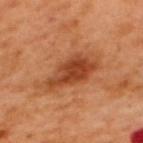Recorded during total-body skin imaging; not selected for excision or biopsy. Longest diameter approximately 7.5 mm. This image is a 15 mm lesion crop taken from a total-body photograph. The tile uses cross-polarized illumination. A male patient, in their 50s. The lesion is located on the upper back.